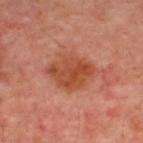Q: Illumination type?
A: cross-polarized
Q: What is the lesion's diameter?
A: about 5.5 mm
Q: Who is the patient?
A: in their mid-50s
Q: Where on the body is the lesion?
A: the upper back
Q: How was this image acquired?
A: ~15 mm crop, total-body skin-cancer survey
Q: Automated lesion metrics?
A: a footprint of about 17 mm² and an eccentricity of roughly 0.65; lesion-presence confidence of about 100/100